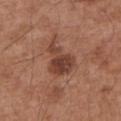Captured during whole-body skin photography for melanoma surveillance; the lesion was not biopsied.
A 15 mm close-up tile from a total-body photography series done for melanoma screening.
On the left upper arm.
A male subject, aged 53–57.